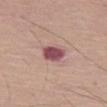Clinical impression: Recorded during total-body skin imaging; not selected for excision or biopsy. Context: The patient is a male aged around 65. A 15 mm close-up extracted from a 3D total-body photography capture. Captured under white-light illumination. Automated tile analysis of the lesion measured an area of roughly 6.5 mm², a shape eccentricity near 0.35, and two-axis asymmetry of about 0.2. The software also gave an average lesion color of about L≈50 a*≈26 b*≈17 (CIELAB), a lesion–skin lightness drop of about 15, and a normalized border contrast of about 11.5. The software also gave border irregularity of about 2 on a 0–10 scale, a within-lesion color-variation index near 2.5/10, and peripheral color asymmetry of about 1. And it measured a detector confidence of about 100 out of 100 that the crop contains a lesion. Measured at roughly 3 mm in maximum diameter. From the left thigh.